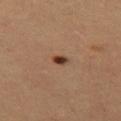| feature | finding |
|---|---|
| workup | no biopsy performed (imaged during a skin exam) |
| acquisition | ~15 mm crop, total-body skin-cancer survey |
| patient | female, approximately 55 years of age |
| body site | the left thigh |
| illumination | cross-polarized |
| TBP lesion metrics | a shape eccentricity near 0.75 and two-axis asymmetry of about 0.35; a lesion color around L≈40 a*≈21 b*≈31 in CIELAB, a lesion–skin lightness drop of about 15, and a normalized lesion–skin contrast near 11.5; a border-irregularity index near 2.5/10 and a within-lesion color-variation index near 2.5/10; an automated nevus-likeness rating near 100 out of 100 and lesion-presence confidence of about 100/100 |
| size | ≈2 mm |